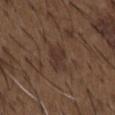  biopsy_status: not biopsied; imaged during a skin examination
  image:
    source: total-body photography crop
    field_of_view_mm: 15
  site: chest
  patient:
    sex: male
    age_approx: 50